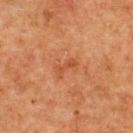| feature | finding |
|---|---|
| biopsy status | total-body-photography surveillance lesion; no biopsy |
| lighting | cross-polarized illumination |
| location | the upper back |
| lesion size | ~2.5 mm (longest diameter) |
| subject | female, about 50 years old |
| image | 15 mm crop, total-body photography |